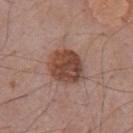Part of a total-body skin-imaging series; this lesion was reviewed on a skin check and was not flagged for biopsy. The subject is a male aged 58–62. Approximately 5 mm at its widest. Captured under white-light illumination. A 15 mm close-up extracted from a 3D total-body photography capture. The lesion is on the front of the torso.